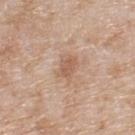Findings:
– biopsy status · no biopsy performed (imaged during a skin exam)
– image · 15 mm crop, total-body photography
– location · the back
– patient · male, aged 78–82
– diameter · ~2.5 mm (longest diameter)